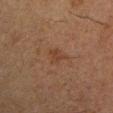biopsy status = total-body-photography surveillance lesion; no biopsy | lesion size = ~3 mm (longest diameter) | acquisition = total-body-photography crop, ~15 mm field of view | tile lighting = cross-polarized illumination | patient = male, in their 50s | body site = the left upper arm.